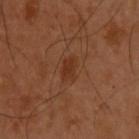– biopsy status · catalogued during a skin exam; not biopsied
– automated metrics · about 6 CIELAB-L* units darker than the surrounding skin; border irregularity of about 2.5 on a 0–10 scale and a color-variation rating of about 1.5/10; an automated nevus-likeness rating near 30 out of 100 and a lesion-detection confidence of about 100/100
– acquisition · ~15 mm crop, total-body skin-cancer survey
– site · the upper back
– subject · male, in their mid- to late 50s